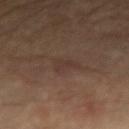Findings:
• workup: catalogued during a skin exam; not biopsied
• image source: ~15 mm tile from a whole-body skin photo
• lighting: cross-polarized
• body site: the right forearm
• lesion size: ~3 mm (longest diameter)
• subject: male, aged 53 to 57